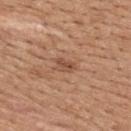biopsy_status: not biopsied; imaged during a skin examination
site: back
patient:
  sex: female
  age_approx: 45
image:
  source: total-body photography crop
  field_of_view_mm: 15
automated_metrics:
  area_mm2_approx: 3.0
  eccentricity: 0.75
  cielab_L: 50
  cielab_a: 22
  cielab_b: 31
  vs_skin_darker_L: 9.0
  vs_skin_contrast_norm: 6.5
  border_irregularity_0_10: 3.0
  color_variation_0_10: 3.5
  peripheral_color_asymmetry: 1.5
  nevus_likeness_0_100: 0
  lesion_detection_confidence_0_100: 100
lighting: white-light